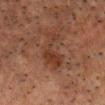Q: Was a biopsy performed?
A: no biopsy performed (imaged during a skin exam)
Q: How large is the lesion?
A: ≈5.5 mm
Q: What did automated image analysis measure?
A: an area of roughly 9.5 mm², an outline eccentricity of about 0.9 (0 = round, 1 = elongated), and two-axis asymmetry of about 0.65; an automated nevus-likeness rating near 0 out of 100 and a lesion-detection confidence of about 100/100
Q: What kind of image is this?
A: total-body-photography crop, ~15 mm field of view
Q: What lighting was used for the tile?
A: cross-polarized
Q: Lesion location?
A: the head or neck
Q: What are the patient's age and sex?
A: male, roughly 60 years of age